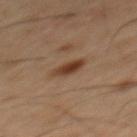follow-up: catalogued during a skin exam; not biopsied